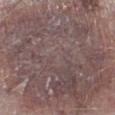{
  "biopsy_status": "not biopsied; imaged during a skin examination"
}